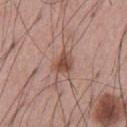{
  "biopsy_status": "not biopsied; imaged during a skin examination",
  "site": "abdomen",
  "lesion_size": {
    "long_diameter_mm_approx": 3.0
  },
  "lighting": "white-light",
  "patient": {
    "sex": "male",
    "age_approx": 55
  },
  "image": {
    "source": "total-body photography crop",
    "field_of_view_mm": 15
  }
}